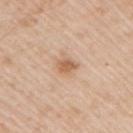Clinical impression: The lesion was tiled from a total-body skin photograph and was not biopsied. Acquisition and patient details: The subject is a male in their 50s. An algorithmic analysis of the crop reported a lesion color around L≈62 a*≈19 b*≈34 in CIELAB, about 10 CIELAB-L* units darker than the surrounding skin, and a normalized lesion–skin contrast near 7. It also reported border irregularity of about 2.5 on a 0–10 scale, a color-variation rating of about 2/10, and a peripheral color-asymmetry measure near 0.5. The software also gave a classifier nevus-likeness of about 55/100 and a detector confidence of about 100 out of 100 that the crop contains a lesion. A region of skin cropped from a whole-body photographic capture, roughly 15 mm wide. About 3 mm across. Captured under white-light illumination. Located on the right upper arm.